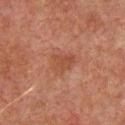Q: Was this lesion biopsied?
A: imaged on a skin check; not biopsied
Q: What is the lesion's diameter?
A: ~3.5 mm (longest diameter)
Q: What is the anatomic site?
A: the front of the torso
Q: How was this image acquired?
A: 15 mm crop, total-body photography
Q: What are the patient's age and sex?
A: male, roughly 60 years of age
Q: How was the tile lit?
A: cross-polarized illumination